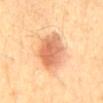follow-up: imaged on a skin check; not biopsied | TBP lesion metrics: a within-lesion color-variation index near 6.5/10 and radial color variation of about 2; lesion-presence confidence of about 100/100 | patient: male, roughly 70 years of age | body site: the mid back | lesion diameter: ≈7 mm | tile lighting: cross-polarized illumination | acquisition: total-body-photography crop, ~15 mm field of view.